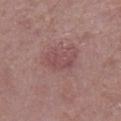subject: male, aged 68–72 | illumination: white-light illumination | anatomic site: the leg | automated metrics: a lesion area of about 6.5 mm² and a symmetry-axis asymmetry near 0.35; a lesion–skin lightness drop of about 7; a border-irregularity index near 4.5/10, a color-variation rating of about 2/10, and radial color variation of about 0.5 | imaging modality: total-body-photography crop, ~15 mm field of view.